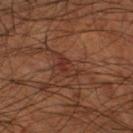Case summary:
- biopsy status · catalogued during a skin exam; not biopsied
- site · the left lower leg
- size · ~3.5 mm (longest diameter)
- acquisition · ~15 mm tile from a whole-body skin photo
- subject · male, approximately 55 years of age
- tile lighting · cross-polarized illumination